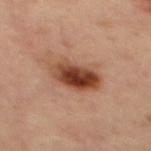biopsy status: imaged on a skin check; not biopsied
imaging modality: total-body-photography crop, ~15 mm field of view
body site: the mid back
patient: female, aged around 45